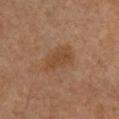The subject is a female about 60 years old.
The lesion is located on the chest.
A 15 mm close-up tile from a total-body photography series done for melanoma screening.